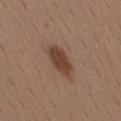Findings:
– follow-up · total-body-photography surveillance lesion; no biopsy
– subject · male, about 40 years old
– image source · 15 mm crop, total-body photography
– image-analysis metrics · a mean CIELAB color near L≈42 a*≈19 b*≈27 and roughly 11 lightness units darker than nearby skin
– body site · the back